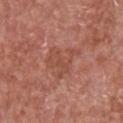Clinical impression: No biopsy was performed on this lesion — it was imaged during a full skin examination and was not determined to be concerning. Acquisition and patient details: The subject is a male about 65 years old. Cropped from a whole-body photographic skin survey; the tile spans about 15 mm. The total-body-photography lesion software estimated a footprint of about 11 mm², an outline eccentricity of about 0.6 (0 = round, 1 = elongated), and a symmetry-axis asymmetry near 0.45. The lesion is on the chest.